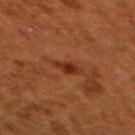workup = total-body-photography surveillance lesion; no biopsy | TBP lesion metrics = an average lesion color of about L≈25 a*≈23 b*≈28 (CIELAB) and about 8 CIELAB-L* units darker than the surrounding skin; a border-irregularity rating of about 2.5/10 and peripheral color asymmetry of about 1 | lesion size = ≈3 mm | location = the back | patient = female, aged 48–52 | acquisition = ~15 mm crop, total-body skin-cancer survey | illumination = cross-polarized illumination.